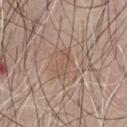Clinical impression: The lesion was photographed on a routine skin check and not biopsied; there is no pathology result. Clinical summary: Located on the chest. Cropped from a total-body skin-imaging series; the visible field is about 15 mm. A male patient aged 63 to 67.